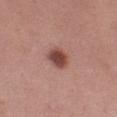follow-up: no biopsy performed (imaged during a skin exam)
TBP lesion metrics: a lesion area of about 5.5 mm² and two-axis asymmetry of about 0.15; a mean CIELAB color near L≈46 a*≈24 b*≈25 and roughly 14 lightness units darker than nearby skin
illumination: white-light
patient: female, aged around 50
body site: the left thigh
imaging modality: total-body-photography crop, ~15 mm field of view